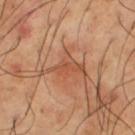No biopsy was performed on this lesion — it was imaged during a full skin examination and was not determined to be concerning. The lesion is located on the left thigh. A lesion tile, about 15 mm wide, cut from a 3D total-body photograph. The tile uses cross-polarized illumination. The recorded lesion diameter is about 5 mm. A male patient, in their mid-60s. Automated image analysis of the tile measured an outline eccentricity of about 0.8 (0 = round, 1 = elongated) and two-axis asymmetry of about 0.55. And it measured a mean CIELAB color near L≈52 a*≈25 b*≈34, roughly 8 lightness units darker than nearby skin, and a normalized border contrast of about 6. The analysis additionally found a border-irregularity index near 7.5/10, a color-variation rating of about 2/10, and peripheral color asymmetry of about 0.5. It also reported a classifier nevus-likeness of about 0/100 and lesion-presence confidence of about 55/100.